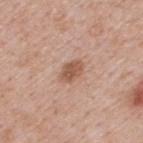Findings:
* follow-up — imaged on a skin check; not biopsied
* patient — male, aged approximately 40
* image — total-body-photography crop, ~15 mm field of view
* lighting — white-light
* anatomic site — the back